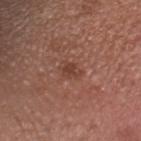{
  "biopsy_status": "not biopsied; imaged during a skin examination",
  "image": {
    "source": "total-body photography crop",
    "field_of_view_mm": 15
  },
  "site": "head or neck",
  "patient": {
    "sex": "male",
    "age_approx": 35
  }
}